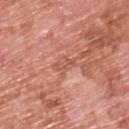Findings:
– workup — imaged on a skin check; not biopsied
– location — the upper back
– subject — male, aged around 70
– image — total-body-photography crop, ~15 mm field of view